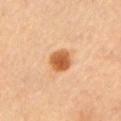Part of a total-body skin-imaging series; this lesion was reviewed on a skin check and was not flagged for biopsy. A male subject aged 63–67. Approximately 3 mm at its widest. Automated image analysis of the tile measured a lesion area of about 6.5 mm² and an outline eccentricity of about 0.25 (0 = round, 1 = elongated). And it measured an average lesion color of about L≈56 a*≈26 b*≈40 (CIELAB), a lesion–skin lightness drop of about 15, and a lesion-to-skin contrast of about 10.5 (normalized; higher = more distinct). The analysis additionally found a within-lesion color-variation index near 4/10 and peripheral color asymmetry of about 1.5. It also reported a nevus-likeness score of about 100/100 and lesion-presence confidence of about 100/100. A 15 mm crop from a total-body photograph taken for skin-cancer surveillance. The lesion is on the left upper arm.